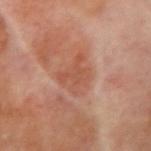The lesion-visualizer software estimated a footprint of about 9.5 mm², an outline eccentricity of about 0.65 (0 = round, 1 = elongated), and two-axis asymmetry of about 0.35. The analysis additionally found internal color variation of about 2.5 on a 0–10 scale. Located on the right upper arm. About 4 mm across. The tile uses cross-polarized illumination. The subject is a male aged 68–72. A 15 mm crop from a total-body photograph taken for skin-cancer surveillance.